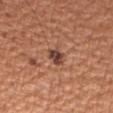Clinical impression: The lesion was tiled from a total-body skin photograph and was not biopsied. Acquisition and patient details: Automated image analysis of the tile measured two-axis asymmetry of about 0.25. The analysis additionally found a border-irregularity rating of about 2/10, internal color variation of about 5 on a 0–10 scale, and peripheral color asymmetry of about 1.5. And it measured a classifier nevus-likeness of about 35/100. The lesion's longest dimension is about 2.5 mm. From the arm. The subject is a male aged approximately 55. A 15 mm close-up extracted from a 3D total-body photography capture. Captured under white-light illumination.